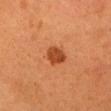| feature | finding |
|---|---|
| image source | total-body-photography crop, ~15 mm field of view |
| tile lighting | cross-polarized illumination |
| subject | female, approximately 55 years of age |
| lesion diameter | ≈2.5 mm |
| location | the head or neck |